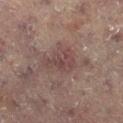biopsy_status: not biopsied; imaged during a skin examination
site: leg
automated_metrics:
  cielab_L: 39
  cielab_a: 17
  cielab_b: 18
  vs_skin_contrast_norm: 6.0
  color_variation_0_10: 3.0
  peripheral_color_asymmetry: 1.0
lesion_size:
  long_diameter_mm_approx: 4.0
patient:
  sex: female
  age_approx: 80
image:
  source: total-body photography crop
  field_of_view_mm: 15
lighting: cross-polarized